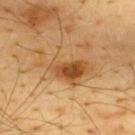biopsy_status: not biopsied; imaged during a skin examination
lighting: cross-polarized
image:
  source: total-body photography crop
  field_of_view_mm: 15
patient:
  sex: male
  age_approx: 65
site: upper back
automated_metrics:
  area_mm2_approx: 17.0
  eccentricity: 0.9
  shape_asymmetry: 0.4
  cielab_L: 55
  cielab_a: 22
  cielab_b: 42
  vs_skin_darker_L: 12.0
  vs_skin_contrast_norm: 8.0